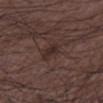Q: Was this lesion biopsied?
A: imaged on a skin check; not biopsied
Q: Who is the patient?
A: male, aged approximately 50
Q: What kind of image is this?
A: ~15 mm crop, total-body skin-cancer survey
Q: What is the anatomic site?
A: the leg